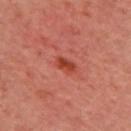Image and clinical context: Longest diameter approximately 2.5 mm. Cropped from a total-body skin-imaging series; the visible field is about 15 mm. Located on the upper back. The tile uses cross-polarized illumination. The patient is a male in their mid-60s.